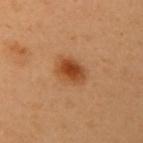The lesion was photographed on a routine skin check and not biopsied; there is no pathology result.
Captured under cross-polarized illumination.
Cropped from a total-body skin-imaging series; the visible field is about 15 mm.
The lesion is on the front of the torso.
The lesion's longest dimension is about 3.5 mm.
A female subject aged around 60.